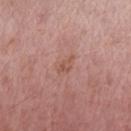| key | value |
|---|---|
| patient | male, aged 53 to 57 |
| lesion size | ≈3 mm |
| lighting | white-light illumination |
| image source | total-body-photography crop, ~15 mm field of view |
| location | the arm |